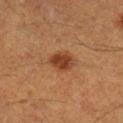Assessment:
Captured during whole-body skin photography for melanoma surveillance; the lesion was not biopsied.
Context:
A roughly 15 mm field-of-view crop from a total-body skin photograph. The lesion's longest dimension is about 3 mm. Imaged with cross-polarized lighting. The lesion is located on the right lower leg. The patient is a male in their 60s.